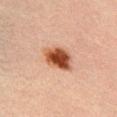About 4 mm across. Located on the chest. Imaged with cross-polarized lighting. The patient is a male aged 28–32. A 15 mm close-up extracted from a 3D total-body photography capture.